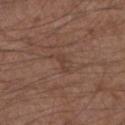The lesion was photographed on a routine skin check and not biopsied; there is no pathology result. The recorded lesion diameter is about 2.5 mm. A region of skin cropped from a whole-body photographic capture, roughly 15 mm wide. A male patient, aged 48–52. The lesion is located on the arm. Captured under white-light illumination.